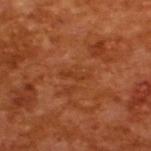biopsy status: total-body-photography surveillance lesion; no biopsy
tile lighting: cross-polarized illumination
lesion diameter: about 3 mm
patient: male, approximately 65 years of age
automated lesion analysis: a lesion color around L≈37 a*≈27 b*≈36 in CIELAB, about 6 CIELAB-L* units darker than the surrounding skin, and a normalized border contrast of about 5
acquisition: total-body-photography crop, ~15 mm field of view